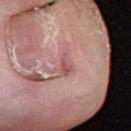<tbp_lesion>
<biopsy_status>not biopsied; imaged during a skin examination</biopsy_status>
<patient>
  <sex>male</sex>
  <age_approx>60</age_approx>
</patient>
<site>right lower leg</site>
<lesion_size>
  <long_diameter_mm_approx>2.5</long_diameter_mm_approx>
</lesion_size>
<image>
  <source>total-body photography crop</source>
  <field_of_view_mm>15</field_of_view_mm>
</image>
</tbp_lesion>